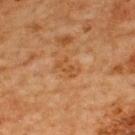follow-up=no biopsy performed (imaged during a skin exam) | size=≈3 mm | acquisition=~15 mm crop, total-body skin-cancer survey | lighting=cross-polarized illumination | TBP lesion metrics=an average lesion color of about L≈44 a*≈21 b*≈36 (CIELAB), a lesion–skin lightness drop of about 6, and a normalized border contrast of about 5.5; border irregularity of about 4.5 on a 0–10 scale, a color-variation rating of about 0/10, and radial color variation of about 0; a nevus-likeness score of about 0/100 and lesion-presence confidence of about 100/100 | patient=male, approximately 65 years of age | anatomic site=the upper back.